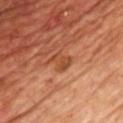Part of a total-body skin-imaging series; this lesion was reviewed on a skin check and was not flagged for biopsy. Located on the chest. A close-up tile cropped from a whole-body skin photograph, about 15 mm across. Measured at roughly 2.5 mm in maximum diameter. The patient is a male aged 68–72. The tile uses cross-polarized illumination.